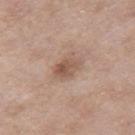Q: Is there a histopathology result?
A: no biopsy performed (imaged during a skin exam)
Q: Automated lesion metrics?
A: a lesion area of about 6.5 mm² and an eccentricity of roughly 0.75; a mean CIELAB color near L≈54 a*≈18 b*≈27 and about 10 CIELAB-L* units darker than the surrounding skin; a within-lesion color-variation index near 5/10; a classifier nevus-likeness of about 15/100 and a lesion-detection confidence of about 100/100
Q: What kind of image is this?
A: total-body-photography crop, ~15 mm field of view
Q: Lesion size?
A: ~3.5 mm (longest diameter)
Q: What is the anatomic site?
A: the right thigh
Q: What are the patient's age and sex?
A: female, roughly 55 years of age
Q: What lighting was used for the tile?
A: white-light